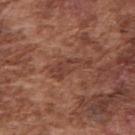Imaged during a routine full-body skin examination; the lesion was not biopsied and no histopathology is available. Approximately 4.5 mm at its widest. This is a white-light tile. Automated image analysis of the tile measured an outline eccentricity of about 0.95 (0 = round, 1 = elongated) and a symmetry-axis asymmetry near 0.6. It also reported a border-irregularity rating of about 8/10, a within-lesion color-variation index near 2/10, and radial color variation of about 0.5. This image is a 15 mm lesion crop taken from a total-body photograph. The lesion is located on the right upper arm. A male patient in their mid-70s.